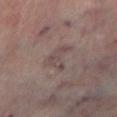Clinical impression:
The lesion was tiled from a total-body skin photograph and was not biopsied.
Acquisition and patient details:
From the left lower leg. A male patient, roughly 65 years of age. A 15 mm crop from a total-body photograph taken for skin-cancer surveillance.